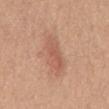{
  "biopsy_status": "not biopsied; imaged during a skin examination",
  "lighting": "white-light",
  "automated_metrics": {
    "cielab_L": 57,
    "cielab_a": 24,
    "cielab_b": 30,
    "vs_skin_darker_L": 8.0,
    "vs_skin_contrast_norm": 5.5
  },
  "patient": {
    "sex": "female",
    "age_approx": 55
  },
  "lesion_size": {
    "long_diameter_mm_approx": 4.5
  },
  "image": {
    "source": "total-body photography crop",
    "field_of_view_mm": 15
  },
  "site": "chest"
}